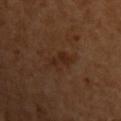Assessment:
Recorded during total-body skin imaging; not selected for excision or biopsy.